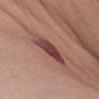Q: What is the imaging modality?
A: total-body-photography crop, ~15 mm field of view
Q: Who is the patient?
A: male, aged 28–32
Q: What is the lesion's diameter?
A: ≈5 mm
Q: Illumination type?
A: white-light illumination